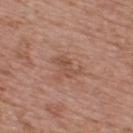Assessment:
Captured during whole-body skin photography for melanoma surveillance; the lesion was not biopsied.
Image and clinical context:
The recorded lesion diameter is about 3 mm. The lesion is on the upper back. An algorithmic analysis of the crop reported a footprint of about 4 mm² and an eccentricity of roughly 0.8. The software also gave border irregularity of about 4.5 on a 0–10 scale and a peripheral color-asymmetry measure near 1. The patient is a male aged approximately 75. This is a white-light tile. A region of skin cropped from a whole-body photographic capture, roughly 15 mm wide.